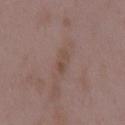Imaged during a routine full-body skin examination; the lesion was not biopsied and no histopathology is available. The subject is a female aged around 35. The tile uses white-light illumination. A 15 mm crop from a total-body photograph taken for skin-cancer surveillance. From the back. The recorded lesion diameter is about 3 mm. Automated image analysis of the tile measured a border-irregularity rating of about 4.5/10, a color-variation rating of about 1/10, and a peripheral color-asymmetry measure near 0.5. And it measured an automated nevus-likeness rating near 0 out of 100 and a lesion-detection confidence of about 100/100.